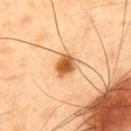Findings:
– biopsy status: catalogued during a skin exam; not biopsied
– imaging modality: ~15 mm tile from a whole-body skin photo
– subject: male, in their 40s
– diameter: about 2.5 mm
– anatomic site: the back
– lighting: cross-polarized illumination